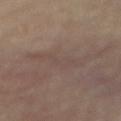Captured during whole-body skin photography for melanoma surveillance; the lesion was not biopsied.
A close-up tile cropped from a whole-body skin photograph, about 15 mm across.
Automated image analysis of the tile measured a normalized border contrast of about 3. The software also gave a border-irregularity rating of about 6/10, a within-lesion color-variation index near 2/10, and radial color variation of about 0.5.
On the mid back.
Captured under cross-polarized illumination.
Approximately 5.5 mm at its widest.
A female patient about 65 years old.